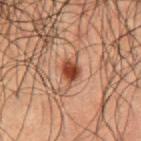This lesion was catalogued during total-body skin photography and was not selected for biopsy. The subject is a male approximately 50 years of age. The tile uses cross-polarized illumination. Approximately 2.5 mm at its widest. A lesion tile, about 15 mm wide, cut from a 3D total-body photograph. The lesion is on the abdomen.